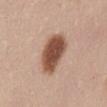The total-body-photography lesion software estimated a lesion color around L≈50 a*≈21 b*≈29 in CIELAB and roughly 18 lightness units darker than nearby skin. It also reported a nevus-likeness score of about 100/100. Captured under white-light illumination. Approximately 5 mm at its widest. A 15 mm close-up tile from a total-body photography series done for melanoma screening. On the abdomen. A female patient aged 48–52.